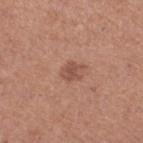Clinical impression:
Recorded during total-body skin imaging; not selected for excision or biopsy.
Background:
The lesion-visualizer software estimated a footprint of about 3.5 mm² and a symmetry-axis asymmetry near 0.3. It also reported a lesion color around L≈51 a*≈23 b*≈28 in CIELAB, about 9 CIELAB-L* units darker than the surrounding skin, and a normalized lesion–skin contrast near 6.5. And it measured border irregularity of about 3.5 on a 0–10 scale, a within-lesion color-variation index near 1.5/10, and peripheral color asymmetry of about 0.5. The subject is a female aged around 40. The lesion's longest dimension is about 2.5 mm. A 15 mm crop from a total-body photograph taken for skin-cancer surveillance. Captured under white-light illumination. The lesion is located on the left lower leg.